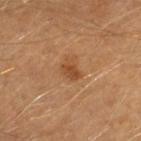  biopsy_status: not biopsied; imaged during a skin examination
  image:
    source: total-body photography crop
    field_of_view_mm: 15
  lesion_size:
    long_diameter_mm_approx: 2.5
  patient:
    sex: male
    age_approx: 40
  automated_metrics:
    area_mm2_approx: 4.0
    eccentricity: 0.75
    shape_asymmetry: 0.3
    nevus_likeness_0_100: 75
    lesion_detection_confidence_0_100: 100
  site: left forearm
  lighting: cross-polarized